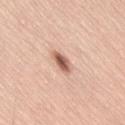Assessment: Part of a total-body skin-imaging series; this lesion was reviewed on a skin check and was not flagged for biopsy. Acquisition and patient details: This image is a 15 mm lesion crop taken from a total-body photograph. This is a white-light tile. A male subject aged 38–42. The lesion is on the lower back. About 3.5 mm across.